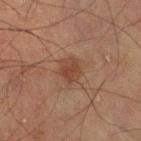Case summary:
- follow-up: total-body-photography surveillance lesion; no biopsy
- image: total-body-photography crop, ~15 mm field of view
- lighting: cross-polarized
- image-analysis metrics: an area of roughly 6.5 mm², a shape eccentricity near 0.55, and a shape-asymmetry score of about 0.2 (0 = symmetric); an average lesion color of about L≈34 a*≈17 b*≈24 (CIELAB); radial color variation of about 1; an automated nevus-likeness rating near 45 out of 100 and a detector confidence of about 100 out of 100 that the crop contains a lesion
- patient: male, aged around 65
- anatomic site: the left thigh
- lesion diameter: ~3 mm (longest diameter)